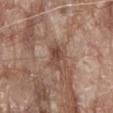Clinical impression:
The lesion was tiled from a total-body skin photograph and was not biopsied.
Clinical summary:
Approximately 3.5 mm at its widest. An algorithmic analysis of the crop reported two-axis asymmetry of about 0.3. The analysis additionally found a nevus-likeness score of about 0/100 and a detector confidence of about 100 out of 100 that the crop contains a lesion. Located on the abdomen. A 15 mm close-up tile from a total-body photography series done for melanoma screening. A male patient aged around 80.